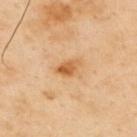imaging modality = ~15 mm tile from a whole-body skin photo; lesion size = about 3 mm; subject = male, in their mid-50s; anatomic site = the back.